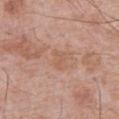The lesion was photographed on a routine skin check and not biopsied; there is no pathology result. The lesion-visualizer software estimated a footprint of about 4 mm² and a shape eccentricity near 0.75. And it measured a mean CIELAB color near L≈58 a*≈21 b*≈30 and about 5 CIELAB-L* units darker than the surrounding skin. It also reported border irregularity of about 3 on a 0–10 scale and peripheral color asymmetry of about 1. A region of skin cropped from a whole-body photographic capture, roughly 15 mm wide. This is a white-light tile. A male subject, aged 68 to 72. The lesion is on the abdomen.